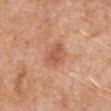biopsy status: imaged on a skin check; not biopsied
subject: male, aged 53 to 57
body site: the chest
image: ~15 mm crop, total-body skin-cancer survey